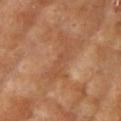| field | value |
|---|---|
| workup | catalogued during a skin exam; not biopsied |
| diameter | ~6 mm (longest diameter) |
| patient | female, aged 73–77 |
| image | 15 mm crop, total-body photography |
| body site | the left upper arm |
| tile lighting | cross-polarized illumination |